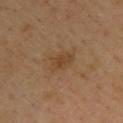Background: Captured under cross-polarized illumination. Cropped from a whole-body photographic skin survey; the tile spans about 15 mm. From the upper back. A male subject, aged approximately 40. The lesion-visualizer software estimated a lesion color around L≈43 a*≈18 b*≈33 in CIELAB and a normalized lesion–skin contrast near 6.